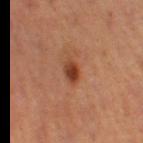  biopsy_status: not biopsied; imaged during a skin examination
  site: right thigh
  image:
    source: total-body photography crop
    field_of_view_mm: 15
  patient:
    sex: female
    age_approx: 50